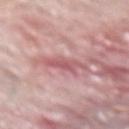Image and clinical context: The lesion is on the right forearm. A region of skin cropped from a whole-body photographic capture, roughly 15 mm wide. The lesion-visualizer software estimated border irregularity of about 4 on a 0–10 scale, a color-variation rating of about 2.5/10, and radial color variation of about 1. The software also gave a classifier nevus-likeness of about 0/100 and a lesion-detection confidence of about 85/100. Imaged with white-light lighting. The recorded lesion diameter is about 4 mm. A male patient aged 73–77.